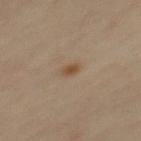<tbp_lesion>
<patient>
  <sex>male</sex>
  <age_approx>55</age_approx>
</patient>
<automated_metrics>
  <vs_skin_darker_L>8.0</vs_skin_darker_L>
  <vs_skin_contrast_norm>7.5</vs_skin_contrast_norm>
  <border_irregularity_0_10>1.5</border_irregularity_0_10>
  <peripheral_color_asymmetry>0.5</peripheral_color_asymmetry>
</automated_metrics>
<lighting>cross-polarized</lighting>
<image>
  <source>total-body photography crop</source>
  <field_of_view_mm>15</field_of_view_mm>
</image>
<lesion_size>
  <long_diameter_mm_approx>2.0</long_diameter_mm_approx>
</lesion_size>
<site>upper back</site>
</tbp_lesion>